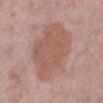Case summary:
* follow-up: catalogued during a skin exam; not biopsied
* illumination: white-light
* size: ≈8 mm
* acquisition: ~15 mm tile from a whole-body skin photo
* automated metrics: a footprint of about 38 mm², an outline eccentricity of about 0.65 (0 = round, 1 = elongated), and two-axis asymmetry of about 0.15; an average lesion color of about L≈56 a*≈20 b*≈25 (CIELAB) and a normalized lesion–skin contrast near 6; a border-irregularity index near 2/10 and radial color variation of about 1
* subject: female, approximately 60 years of age
* body site: the left lower leg